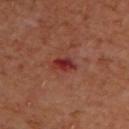Q: Is there a histopathology result?
A: total-body-photography surveillance lesion; no biopsy
Q: What is the anatomic site?
A: the upper back
Q: What lighting was used for the tile?
A: cross-polarized illumination
Q: How was this image acquired?
A: total-body-photography crop, ~15 mm field of view
Q: Lesion size?
A: about 3 mm
Q: Patient demographics?
A: male, about 70 years old
Q: Automated lesion metrics?
A: a border-irregularity rating of about 3/10 and radial color variation of about 1; a classifier nevus-likeness of about 0/100 and a lesion-detection confidence of about 100/100